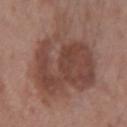A female subject roughly 55 years of age.
A 15 mm close-up tile from a total-body photography series done for melanoma screening.
Measured at roughly 10.5 mm in maximum diameter.
On the left forearm.
The tile uses white-light illumination.